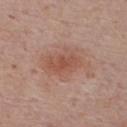Imaged during a routine full-body skin examination; the lesion was not biopsied and no histopathology is available. The lesion is on the chest. Captured under white-light illumination. A 15 mm crop from a total-body photograph taken for skin-cancer surveillance. A male subject aged approximately 70.